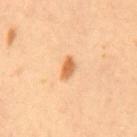biopsy status: imaged on a skin check; not biopsied
patient: female, about 50 years old
image source: ~15 mm crop, total-body skin-cancer survey
size: ≈3 mm
illumination: cross-polarized